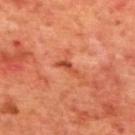The lesion was tiled from a total-body skin photograph and was not biopsied.
On the mid back.
The tile uses cross-polarized illumination.
A male subject approximately 70 years of age.
A close-up tile cropped from a whole-body skin photograph, about 15 mm across.